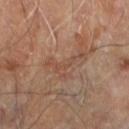<tbp_lesion>
<biopsy_status>not biopsied; imaged during a skin examination</biopsy_status>
<site>left lower leg</site>
<image>
  <source>total-body photography crop</source>
  <field_of_view_mm>15</field_of_view_mm>
</image>
<patient>
  <sex>male</sex>
  <age_approx>70</age_approx>
</patient>
</tbp_lesion>